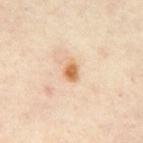This lesion was catalogued during total-body skin photography and was not selected for biopsy. The recorded lesion diameter is about 2.5 mm. The patient is approximately 55 years of age. On the abdomen. Automated image analysis of the tile measured a mean CIELAB color near L≈66 a*≈20 b*≈38, roughly 12 lightness units darker than nearby skin, and a lesion-to-skin contrast of about 9 (normalized; higher = more distinct). It also reported a border-irregularity rating of about 2.5/10 and internal color variation of about 3 on a 0–10 scale. Cropped from a total-body skin-imaging series; the visible field is about 15 mm.